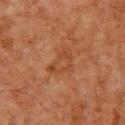| feature | finding |
|---|---|
| biopsy status | catalogued during a skin exam; not biopsied |
| anatomic site | the upper back |
| patient | male, aged approximately 60 |
| tile lighting | cross-polarized |
| acquisition | total-body-photography crop, ~15 mm field of view |
| automated metrics | an outline eccentricity of about 0.75 (0 = round, 1 = elongated) and two-axis asymmetry of about 0.55; a lesion color around L≈41 a*≈23 b*≈34 in CIELAB, a lesion–skin lightness drop of about 6, and a normalized border contrast of about 5.5; a border-irregularity rating of about 9/10, a color-variation rating of about 0.5/10, and radial color variation of about 0; a nevus-likeness score of about 0/100 and a detector confidence of about 100 out of 100 that the crop contains a lesion |
| lesion size | ~4 mm (longest diameter) |